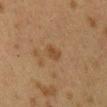follow-up: no biopsy performed (imaged during a skin exam)
patient: female, roughly 40 years of age
image: ~15 mm crop, total-body skin-cancer survey
site: the mid back
lesion size: about 2.5 mm
lighting: cross-polarized illumination